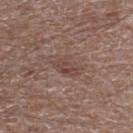biopsy status — catalogued during a skin exam; not biopsied | imaging modality — 15 mm crop, total-body photography | lighting — white-light | patient — male, in their 70s | body site — the right lower leg | lesion size — ≈3 mm.